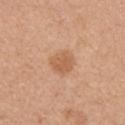- notes: no biopsy performed (imaged during a skin exam)
- imaging modality: 15 mm crop, total-body photography
- site: the left upper arm
- patient: female, approximately 35 years of age